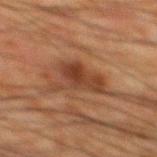Assessment:
Part of a total-body skin-imaging series; this lesion was reviewed on a skin check and was not flagged for biopsy.
Acquisition and patient details:
A roughly 15 mm field-of-view crop from a total-body skin photograph. The lesion is on the mid back. The patient is a male aged 63–67.